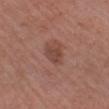Impression:
Imaged during a routine full-body skin examination; the lesion was not biopsied and no histopathology is available.
Clinical summary:
From the arm. Automated image analysis of the tile measured a border-irregularity rating of about 2.5/10, a within-lesion color-variation index near 1.5/10, and radial color variation of about 0.5. It also reported a classifier nevus-likeness of about 15/100. The tile uses white-light illumination. A male patient aged around 60. The recorded lesion diameter is about 3 mm. A 15 mm close-up tile from a total-body photography series done for melanoma screening.